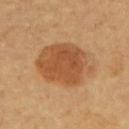<record>
<biopsy_status>not biopsied; imaged during a skin examination</biopsy_status>
<image>
  <source>total-body photography crop</source>
  <field_of_view_mm>15</field_of_view_mm>
</image>
<lesion_size>
  <long_diameter_mm_approx>7.0</long_diameter_mm_approx>
</lesion_size>
<lighting>cross-polarized</lighting>
<site>upper back</site>
<patient>
  <age_approx>60</age_approx>
</patient>
<automated_metrics>
  <border_irregularity_0_10>1.5</border_irregularity_0_10>
  <color_variation_0_10>3.5</color_variation_0_10>
  <peripheral_color_asymmetry>1.0</peripheral_color_asymmetry>
</automated_metrics>
</record>